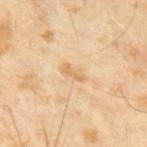Assessment: Captured during whole-body skin photography for melanoma surveillance; the lesion was not biopsied. Background: A male subject in their mid- to late 60s. A roughly 15 mm field-of-view crop from a total-body skin photograph.